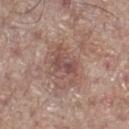No biopsy was performed on this lesion — it was imaged during a full skin examination and was not determined to be concerning.
A 15 mm crop from a total-body photograph taken for skin-cancer surveillance.
A male patient in their 70s.
Imaged with white-light lighting.
The recorded lesion diameter is about 4.5 mm.
The lesion is located on the right lower leg.
An algorithmic analysis of the crop reported about 9 CIELAB-L* units darker than the surrounding skin and a lesion-to-skin contrast of about 6.5 (normalized; higher = more distinct). The software also gave a border-irregularity rating of about 4.5/10 and internal color variation of about 5 on a 0–10 scale. The analysis additionally found an automated nevus-likeness rating near 0 out of 100 and lesion-presence confidence of about 100/100.